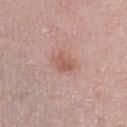{"biopsy_status": "not biopsied; imaged during a skin examination", "site": "left lower leg", "image": {"source": "total-body photography crop", "field_of_view_mm": 15}, "patient": {"sex": "female", "age_approx": 55}, "lighting": "white-light", "lesion_size": {"long_diameter_mm_approx": 2.5}}